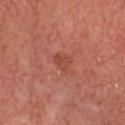biopsy_status: not biopsied; imaged during a skin examination
patient:
  sex: male
  age_approx: 65
automated_metrics:
  color_variation_0_10: 1.5
  peripheral_color_asymmetry: 0.5
  nevus_likeness_0_100: 0
  lesion_detection_confidence_0_100: 100
image:
  source: total-body photography crop
  field_of_view_mm: 15
lighting: cross-polarized
site: head or neck
lesion_size:
  long_diameter_mm_approx: 2.5